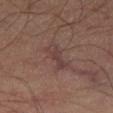Recorded during total-body skin imaging; not selected for excision or biopsy.
The subject is a male aged 63–67.
The lesion is located on the right thigh.
A 15 mm close-up tile from a total-body photography series done for melanoma screening.